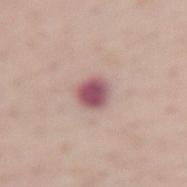<record>
  <biopsy_status>not biopsied; imaged during a skin examination</biopsy_status>
  <site>lower back</site>
  <lesion_size>
    <long_diameter_mm_approx>2.5</long_diameter_mm_approx>
  </lesion_size>
  <automated_metrics>
    <area_mm2_approx>6.0</area_mm2_approx>
    <eccentricity>0.4</eccentricity>
    <nevus_likeness_0_100>0</nevus_likeness_0_100>
    <lesion_detection_confidence_0_100>100</lesion_detection_confidence_0_100>
  </automated_metrics>
  <image>
    <source>total-body photography crop</source>
    <field_of_view_mm>15</field_of_view_mm>
  </image>
  <patient>
    <sex>female</sex>
    <age_approx>75</age_approx>
  </patient>
</record>